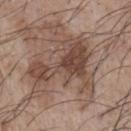Q: Was a biopsy performed?
A: imaged on a skin check; not biopsied
Q: What is the anatomic site?
A: the chest
Q: What did automated image analysis measure?
A: a footprint of about 26 mm², an outline eccentricity of about 0.75 (0 = round, 1 = elongated), and a shape-asymmetry score of about 0.7 (0 = symmetric); a mean CIELAB color near L≈46 a*≈17 b*≈24, roughly 10 lightness units darker than nearby skin, and a normalized border contrast of about 7.5; a border-irregularity rating of about 10/10
Q: Illumination type?
A: white-light illumination
Q: What kind of image is this?
A: 15 mm crop, total-body photography
Q: Who is the patient?
A: male, aged 73 to 77
Q: What is the lesion's diameter?
A: ≈8 mm